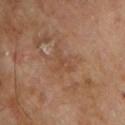The lesion was tiled from a total-body skin photograph and was not biopsied. A 15 mm crop from a total-body photograph taken for skin-cancer surveillance. Located on the left upper arm. Imaged with cross-polarized lighting. A male subject about 70 years old.